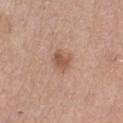The lesion was tiled from a total-body skin photograph and was not biopsied.
The lesion is located on the right lower leg.
A roughly 15 mm field-of-view crop from a total-body skin photograph.
A female subject aged approximately 55.
Approximately 3 mm at its widest.
Imaged with white-light lighting.